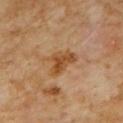Case summary:
* workup — total-body-photography surveillance lesion; no biopsy
* lesion diameter — ≈4 mm
* body site — the front of the torso
* tile lighting — cross-polarized illumination
* patient — male, aged approximately 60
* image — total-body-photography crop, ~15 mm field of view
* automated metrics — a footprint of about 8 mm² and an outline eccentricity of about 0.75 (0 = round, 1 = elongated); a border-irregularity index near 5/10, internal color variation of about 4 on a 0–10 scale, and radial color variation of about 1.5